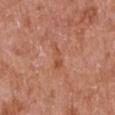* workup — catalogued during a skin exam; not biopsied
* patient — male, about 65 years old
* diameter — about 3.5 mm
* automated lesion analysis — a footprint of about 3.5 mm², an outline eccentricity of about 0.95 (0 = round, 1 = elongated), and a shape-asymmetry score of about 0.5 (0 = symmetric); a mean CIELAB color near L≈52 a*≈27 b*≈34, roughly 7 lightness units darker than nearby skin, and a lesion-to-skin contrast of about 5.5 (normalized; higher = more distinct); a border-irregularity index near 6/10, a within-lesion color-variation index near 0/10, and peripheral color asymmetry of about 0
* body site — the left upper arm
* image source — 15 mm crop, total-body photography
* lighting — white-light